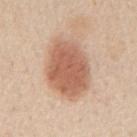patient: male, aged 58 to 62
size: ~7 mm (longest diameter)
tile lighting: white-light
acquisition: total-body-photography crop, ~15 mm field of view
site: the mid back